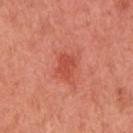– workup — total-body-photography surveillance lesion; no biopsy
– image-analysis metrics — a lesion area of about 5 mm², an outline eccentricity of about 0.6 (0 = round, 1 = elongated), and two-axis asymmetry of about 0.3; an average lesion color of about L≈52 a*≈36 b*≈35 (CIELAB) and roughly 9 lightness units darker than nearby skin; a border-irregularity rating of about 3/10, a within-lesion color-variation index near 1.5/10, and a peripheral color-asymmetry measure near 0.5
– illumination — white-light illumination
– patient — female, aged 48–52
– body site — the right upper arm
– image source — ~15 mm tile from a whole-body skin photo